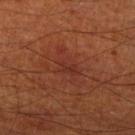Case summary:
– biopsy status — no biopsy performed (imaged during a skin exam)
– subject — male, aged 68 to 72
– illumination — cross-polarized illumination
– image — ~15 mm crop, total-body skin-cancer survey
– site — the right thigh